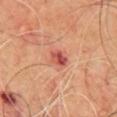Assessment:
The lesion was tiled from a total-body skin photograph and was not biopsied.
Image and clinical context:
Automated tile analysis of the lesion measured a footprint of about 3.5 mm², a shape eccentricity near 0.7, and a shape-asymmetry score of about 0.2 (0 = symmetric). The analysis additionally found a mean CIELAB color near L≈51 a*≈33 b*≈29, roughly 13 lightness units darker than nearby skin, and a lesion-to-skin contrast of about 8.5 (normalized; higher = more distinct). The software also gave a classifier nevus-likeness of about 0/100 and a lesion-detection confidence of about 100/100. Measured at roughly 2.5 mm in maximum diameter. A close-up tile cropped from a whole-body skin photograph, about 15 mm across. The lesion is on the chest. Captured under cross-polarized illumination. The subject is a male aged around 70.